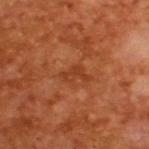biopsy status = no biopsy performed (imaged during a skin exam) | lighting = cross-polarized illumination | patient = male, about 65 years old | lesion size = ≈3.5 mm | acquisition = ~15 mm tile from a whole-body skin photo.